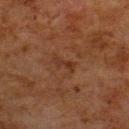| key | value |
|---|---|
| biopsy status | catalogued during a skin exam; not biopsied |
| subject | male, about 80 years old |
| lesion size | ≈2.5 mm |
| body site | the upper back |
| image | ~15 mm tile from a whole-body skin photo |